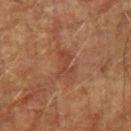workup = no biopsy performed (imaged during a skin exam); image-analysis metrics = a lesion area of about 6 mm², a shape eccentricity near 0.85, and a symmetry-axis asymmetry near 0.35; imaging modality = ~15 mm crop, total-body skin-cancer survey; tile lighting = cross-polarized; body site = the arm; patient = male, in their mid-70s; lesion size = ~3.5 mm (longest diameter).